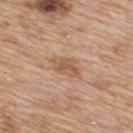{
  "biopsy_status": "not biopsied; imaged during a skin examination",
  "lesion_size": {
    "long_diameter_mm_approx": 3.5
  },
  "patient": {
    "sex": "female",
    "age_approx": 40
  },
  "lighting": "white-light",
  "site": "upper back",
  "image": {
    "source": "total-body photography crop",
    "field_of_view_mm": 15
  }
}